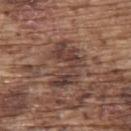{
  "site": "upper back",
  "patient": {
    "sex": "male",
    "age_approx": 75
  },
  "automated_metrics": {
    "vs_skin_contrast_norm": 8.0,
    "border_irregularity_0_10": 7.0,
    "color_variation_0_10": 5.0,
    "peripheral_color_asymmetry": 1.5
  },
  "image": {
    "source": "total-body photography crop",
    "field_of_view_mm": 15
  }
}